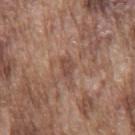follow-up — no biopsy performed (imaged during a skin exam)
imaging modality — ~15 mm tile from a whole-body skin photo
lighting — white-light
size — ~2.5 mm (longest diameter)
patient — male, in their mid-70s
body site — the mid back